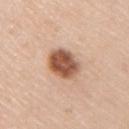Part of a total-body skin-imaging series; this lesion was reviewed on a skin check and was not flagged for biopsy.
A male subject, aged 43 to 47.
A 15 mm crop from a total-body photograph taken for skin-cancer surveillance.
The lesion is located on the right upper arm.